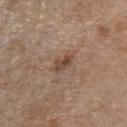Assessment:
The lesion was tiled from a total-body skin photograph and was not biopsied.